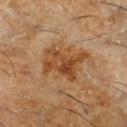biopsy_status: not biopsied; imaged during a skin examination
site: right lower leg
patient:
  sex: male
  age_approx: 60
image:
  source: total-body photography crop
  field_of_view_mm: 15